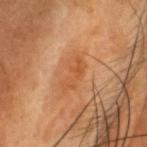patient: male, about 60 years old | acquisition: total-body-photography crop, ~15 mm field of view | body site: the head or neck | automated metrics: a footprint of about 7 mm², an eccentricity of roughly 0.9, and a shape-asymmetry score of about 0.25 (0 = symmetric); a border-irregularity rating of about 3.5/10, internal color variation of about 3 on a 0–10 scale, and a peripheral color-asymmetry measure near 1 | illumination: cross-polarized.